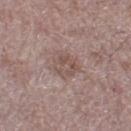<case>
<biopsy_status>not biopsied; imaged during a skin examination</biopsy_status>
<patient>
  <sex>male</sex>
  <age_approx>70</age_approx>
</patient>
<site>left thigh</site>
<lesion_size>
  <long_diameter_mm_approx>3.0</long_diameter_mm_approx>
</lesion_size>
<automated_metrics>
  <area_mm2_approx>6.5</area_mm2_approx>
  <eccentricity>0.35</eccentricity>
  <shape_asymmetry>0.25</shape_asymmetry>
</automated_metrics>
<image>
  <source>total-body photography crop</source>
  <field_of_view_mm>15</field_of_view_mm>
</image>
</case>